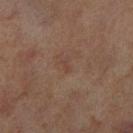Findings:
– notes: catalogued during a skin exam; not biopsied
– subject: male, aged 68 to 72
– illumination: cross-polarized
– site: the right lower leg
– acquisition: 15 mm crop, total-body photography
– size: ≈1 mm